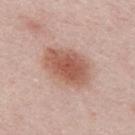Recorded during total-body skin imaging; not selected for excision or biopsy. A roughly 15 mm field-of-view crop from a total-body skin photograph. A male subject approximately 25 years of age. On the mid back. Captured under white-light illumination. About 6 mm across.